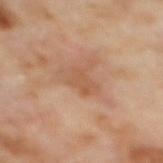Clinical impression:
Recorded during total-body skin imaging; not selected for excision or biopsy.
Context:
The lesion is on the upper back. A roughly 15 mm field-of-view crop from a total-body skin photograph. A female subject aged 58–62. The tile uses cross-polarized illumination.